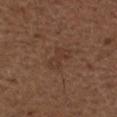biopsy status: catalogued during a skin exam; not biopsied
automated lesion analysis: a footprint of about 5 mm² and two-axis asymmetry of about 0.35; border irregularity of about 4.5 on a 0–10 scale and radial color variation of about 1.5; lesion-presence confidence of about 100/100
patient: male, aged 48–52
lighting: white-light illumination
location: the left upper arm
image: ~15 mm crop, total-body skin-cancer survey